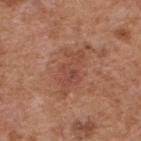Impression:
Captured during whole-body skin photography for melanoma surveillance; the lesion was not biopsied.
Acquisition and patient details:
A roughly 15 mm field-of-view crop from a total-body skin photograph. The lesion is on the upper back. The patient is a male roughly 65 years of age.